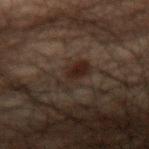Assessment: This lesion was catalogued during total-body skin photography and was not selected for biopsy. Clinical summary: An algorithmic analysis of the crop reported an area of roughly 6 mm² and a shape-asymmetry score of about 0.35 (0 = symmetric). The software also gave a mean CIELAB color near L≈17 a*≈10 b*≈16, roughly 5 lightness units darker than nearby skin, and a normalized lesion–skin contrast near 8. The analysis additionally found border irregularity of about 4.5 on a 0–10 scale and a peripheral color-asymmetry measure near 1.5. The tile uses cross-polarized illumination. Cropped from a total-body skin-imaging series; the visible field is about 15 mm. On the left forearm. A male subject, aged 48–52.